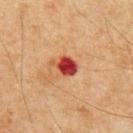Clinical impression:
Part of a total-body skin-imaging series; this lesion was reviewed on a skin check and was not flagged for biopsy.
Image and clinical context:
Located on the chest. The lesion's longest dimension is about 2.5 mm. The tile uses cross-polarized illumination. Cropped from a whole-body photographic skin survey; the tile spans about 15 mm. The subject is a male approximately 65 years of age.